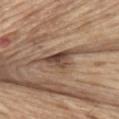{"image": {"source": "total-body photography crop", "field_of_view_mm": 15}, "automated_metrics": {"peripheral_color_asymmetry": 2.0, "nevus_likeness_0_100": 25, "lesion_detection_confidence_0_100": 50}, "patient": {"sex": "male", "age_approx": 70}, "lesion_size": {"long_diameter_mm_approx": 4.0}, "lighting": "white-light", "site": "abdomen"}